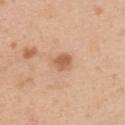Q: Was a biopsy performed?
A: imaged on a skin check; not biopsied
Q: What did automated image analysis measure?
A: a footprint of about 4 mm², an eccentricity of roughly 0.7, and a shape-asymmetry score of about 0.25 (0 = symmetric); a lesion-detection confidence of about 100/100
Q: How was this image acquired?
A: 15 mm crop, total-body photography
Q: What is the anatomic site?
A: the upper back
Q: Illumination type?
A: white-light
Q: What are the patient's age and sex?
A: male, aged approximately 35
Q: How large is the lesion?
A: ≈2.5 mm